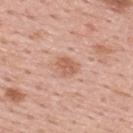This lesion was catalogued during total-body skin photography and was not selected for biopsy. Automated tile analysis of the lesion measured a lesion color around L≈60 a*≈23 b*≈30 in CIELAB and a normalized lesion–skin contrast near 6.5. It also reported lesion-presence confidence of about 100/100. A male patient aged approximately 55. On the upper back. This is a white-light tile. A region of skin cropped from a whole-body photographic capture, roughly 15 mm wide.